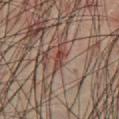Imaged during a routine full-body skin examination; the lesion was not biopsied and no histopathology is available.
Imaged with cross-polarized lighting.
A 15 mm close-up extracted from a 3D total-body photography capture.
A male subject aged 58 to 62.
The lesion is located on the abdomen.
The lesion's longest dimension is about 2.5 mm.
The total-body-photography lesion software estimated a footprint of about 3 mm² and a shape-asymmetry score of about 0.25 (0 = symmetric). It also reported a mean CIELAB color near L≈37 a*≈20 b*≈23 and a normalized border contrast of about 8. It also reported an automated nevus-likeness rating near 35 out of 100 and a lesion-detection confidence of about 60/100.